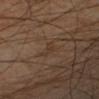Part of a total-body skin-imaging series; this lesion was reviewed on a skin check and was not flagged for biopsy.
A 15 mm close-up extracted from a 3D total-body photography capture.
Located on the left forearm.
The subject is a male aged 48 to 52.
Captured under cross-polarized illumination.